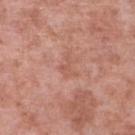Impression: The lesion was tiled from a total-body skin photograph and was not biopsied. Acquisition and patient details: Cropped from a whole-body photographic skin survey; the tile spans about 15 mm. Automated tile analysis of the lesion measured an eccentricity of roughly 0.75 and two-axis asymmetry of about 0.55. The software also gave an average lesion color of about L≈56 a*≈24 b*≈29 (CIELAB) and about 6 CIELAB-L* units darker than the surrounding skin. The analysis additionally found a border-irregularity rating of about 6/10, a within-lesion color-variation index near 0.5/10, and radial color variation of about 0. The software also gave a classifier nevus-likeness of about 0/100 and a lesion-detection confidence of about 100/100. On the leg. Captured under white-light illumination. The patient is a male in their mid-50s.